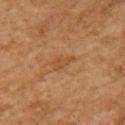Recorded during total-body skin imaging; not selected for excision or biopsy. A region of skin cropped from a whole-body photographic capture, roughly 15 mm wide. About 3 mm across. The lesion is on the upper back. Automated tile analysis of the lesion measured a lesion area of about 3.5 mm², an eccentricity of roughly 0.85, and a shape-asymmetry score of about 0.4 (0 = symmetric). And it measured a mean CIELAB color near L≈43 a*≈19 b*≈34, about 6 CIELAB-L* units darker than the surrounding skin, and a normalized border contrast of about 5. The software also gave a color-variation rating of about 1/10 and radial color variation of about 0.5. It also reported a classifier nevus-likeness of about 0/100. This is a cross-polarized tile. The subject is a female aged 58 to 62.